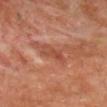Impression: No biopsy was performed on this lesion — it was imaged during a full skin examination and was not determined to be concerning. Clinical summary: A male patient, approximately 65 years of age. From the chest. Captured under cross-polarized illumination. A region of skin cropped from a whole-body photographic capture, roughly 15 mm wide. The recorded lesion diameter is about 4 mm.